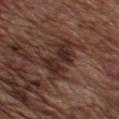A close-up tile cropped from a whole-body skin photograph, about 15 mm across.
The lesion is on the chest.
A male patient, in their mid-70s.